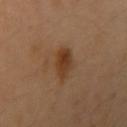  biopsy_status: not biopsied; imaged during a skin examination
  site: right upper arm
  lesion_size:
    long_diameter_mm_approx: 3.0
  lighting: cross-polarized
  image:
    source: total-body photography crop
    field_of_view_mm: 15
  automated_metrics:
    vs_skin_darker_L: 9.0
    vs_skin_contrast_norm: 8.5
    color_variation_0_10: 4.5
    peripheral_color_asymmetry: 1.5
    lesion_detection_confidence_0_100: 100
  patient:
    sex: male
    age_approx: 40